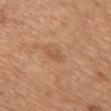biopsy status = no biopsy performed (imaged during a skin exam); image source = ~15 mm tile from a whole-body skin photo; lighting = white-light; subject = female, about 55 years old; anatomic site = the chest; size = about 2.5 mm; automated lesion analysis = a border-irregularity index near 3/10 and internal color variation of about 1 on a 0–10 scale.